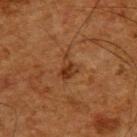follow-up: catalogued during a skin exam; not biopsied | lesion diameter: ~3.5 mm (longest diameter) | TBP lesion metrics: a mean CIELAB color near L≈29 a*≈19 b*≈29, roughly 7 lightness units darker than nearby skin, and a normalized border contrast of about 7.5; a border-irregularity index near 4/10 and internal color variation of about 3 on a 0–10 scale; an automated nevus-likeness rating near 5 out of 100 and a lesion-detection confidence of about 100/100 | patient: male, about 65 years old | acquisition: 15 mm crop, total-body photography | tile lighting: cross-polarized illumination | site: the upper back.